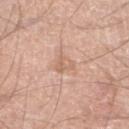Case summary:
* workup · total-body-photography surveillance lesion; no biopsy
* imaging modality · ~15 mm tile from a whole-body skin photo
* site · the left lower leg
* image-analysis metrics · a mean CIELAB color near L≈63 a*≈20 b*≈31 and roughly 7 lightness units darker than nearby skin; a classifier nevus-likeness of about 0/100 and lesion-presence confidence of about 100/100
* illumination · white-light
* subject · male, aged around 60